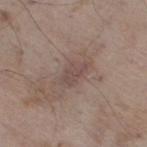Q: Is there a histopathology result?
A: no biopsy performed (imaged during a skin exam)
Q: How was this image acquired?
A: ~15 mm crop, total-body skin-cancer survey
Q: Lesion size?
A: about 3.5 mm
Q: Patient demographics?
A: male, approximately 60 years of age
Q: Where on the body is the lesion?
A: the left lower leg
Q: What did automated image analysis measure?
A: an area of roughly 5 mm²; border irregularity of about 4 on a 0–10 scale and a within-lesion color-variation index near 1.5/10; a detector confidence of about 100 out of 100 that the crop contains a lesion